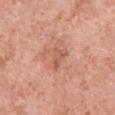The lesion was photographed on a routine skin check and not biopsied; there is no pathology result. On the front of the torso. The subject is a male about 60 years old. Longest diameter approximately 2.5 mm. Automated image analysis of the tile measured border irregularity of about 3.5 on a 0–10 scale and a color-variation rating of about 0.5/10. And it measured a nevus-likeness score of about 0/100. Captured under white-light illumination. A lesion tile, about 15 mm wide, cut from a 3D total-body photograph.